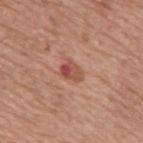body site = the mid back | imaging modality = ~15 mm crop, total-body skin-cancer survey | patient = male, roughly 75 years of age.